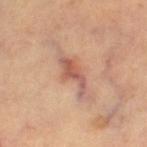{
  "biopsy_status": "not biopsied; imaged during a skin examination",
  "site": "left thigh",
  "image": {
    "source": "total-body photography crop",
    "field_of_view_mm": 15
  },
  "lesion_size": {
    "long_diameter_mm_approx": 4.5
  },
  "lighting": "cross-polarized",
  "patient": {
    "age_approx": 60
  }
}